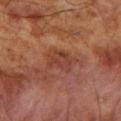Part of a total-body skin-imaging series; this lesion was reviewed on a skin check and was not flagged for biopsy. The subject is a male aged 53 to 57. The lesion-visualizer software estimated an average lesion color of about L≈41 a*≈26 b*≈30 (CIELAB), about 7 CIELAB-L* units darker than the surrounding skin, and a normalized lesion–skin contrast near 6. And it measured a border-irregularity index near 4/10. On the right upper arm. A region of skin cropped from a whole-body photographic capture, roughly 15 mm wide. The lesion's longest dimension is about 3 mm. Imaged with cross-polarized lighting.